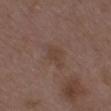Case summary:
– notes: imaged on a skin check; not biopsied
– tile lighting: white-light
– anatomic site: the arm
– patient: female, approximately 30 years of age
– automated metrics: an area of roughly 5 mm², a shape eccentricity near 0.75, and two-axis asymmetry of about 0.3; a mean CIELAB color near L≈40 a*≈16 b*≈24, roughly 5 lightness units darker than nearby skin, and a normalized lesion–skin contrast near 5
– diameter: about 3.5 mm
– imaging modality: total-body-photography crop, ~15 mm field of view